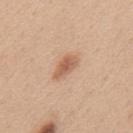This lesion was catalogued during total-body skin photography and was not selected for biopsy. Captured under white-light illumination. The patient is a female aged around 40. From the mid back. Cropped from a whole-body photographic skin survey; the tile spans about 15 mm.